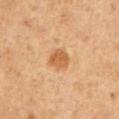Impression: This lesion was catalogued during total-body skin photography and was not selected for biopsy. Image and clinical context: A male subject, approximately 50 years of age. A 15 mm close-up tile from a total-body photography series done for melanoma screening. The lesion is located on the chest.